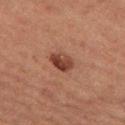Findings:
- follow-up — catalogued during a skin exam; not biopsied
- diameter — ~3 mm (longest diameter)
- site — the left thigh
- patient — female, approximately 60 years of age
- tile lighting — cross-polarized illumination
- image source — ~15 mm tile from a whole-body skin photo
- TBP lesion metrics — a lesion area of about 5.5 mm² and a symmetry-axis asymmetry near 0.25; a lesion–skin lightness drop of about 10 and a normalized lesion–skin contrast near 9.5; a lesion-detection confidence of about 100/100